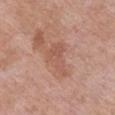Recorded during total-body skin imaging; not selected for excision or biopsy. Automated image analysis of the tile measured a lesion area of about 7 mm², an eccentricity of roughly 0.85, and two-axis asymmetry of about 0.55. The software also gave a mean CIELAB color near L≈55 a*≈22 b*≈29, about 7 CIELAB-L* units darker than the surrounding skin, and a lesion-to-skin contrast of about 5.5 (normalized; higher = more distinct). The subject is a female approximately 70 years of age. The lesion's longest dimension is about 4.5 mm. Captured under white-light illumination. A roughly 15 mm field-of-view crop from a total-body skin photograph. Located on the chest.